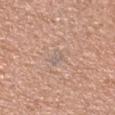Clinical impression:
Recorded during total-body skin imaging; not selected for excision or biopsy.
Background:
A male patient aged 38 to 42. Longest diameter approximately 2.5 mm. This is a white-light tile. This image is a 15 mm lesion crop taken from a total-body photograph. The lesion is located on the head or neck.